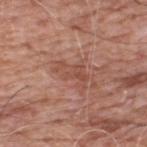<record>
<biopsy_status>not biopsied; imaged during a skin examination</biopsy_status>
<patient>
  <sex>male</sex>
  <age_approx>60</age_approx>
</patient>
<image>
  <source>total-body photography crop</source>
  <field_of_view_mm>15</field_of_view_mm>
</image>
<site>upper back</site>
<lighting>white-light</lighting>
<automated_metrics>
  <area_mm2_approx>6.0</area_mm2_approx>
  <eccentricity>0.85</eccentricity>
  <shape_asymmetry>0.4</shape_asymmetry>
  <border_irregularity_0_10>5.5</border_irregularity_0_10>
  <color_variation_0_10>2.5</color_variation_0_10>
  <peripheral_color_asymmetry>1.0</peripheral_color_asymmetry>
</automated_metrics>
</record>